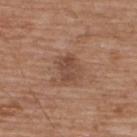patient: male, aged 63 to 67
automated lesion analysis: a nevus-likeness score of about 5/100 and lesion-presence confidence of about 100/100
body site: the upper back
lesion size: about 3 mm
image: ~15 mm tile from a whole-body skin photo